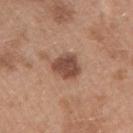Impression:
This lesion was catalogued during total-body skin photography and was not selected for biopsy.
Context:
Approximately 3.5 mm at its widest. The subject is a female roughly 40 years of age. This image is a 15 mm lesion crop taken from a total-body photograph. The tile uses white-light illumination. The lesion is located on the arm.